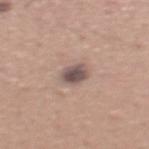biopsy status — total-body-photography surveillance lesion; no biopsy | diameter — about 2.5 mm | body site — the mid back | subject — male, in their mid-40s | image source — total-body-photography crop, ~15 mm field of view | lighting — white-light | image-analysis metrics — a lesion color around L≈51 a*≈15 b*≈19 in CIELAB and a normalized border contrast of about 10.5; a classifier nevus-likeness of about 65/100 and a detector confidence of about 100 out of 100 that the crop contains a lesion.